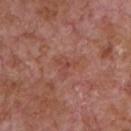Part of a total-body skin-imaging series; this lesion was reviewed on a skin check and was not flagged for biopsy. A male patient in their mid-60s. On the chest. Imaged with white-light lighting. A region of skin cropped from a whole-body photographic capture, roughly 15 mm wide. The recorded lesion diameter is about 3 mm.